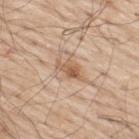– illumination · white-light
– site · the upper back
– patient · male, in their 70s
– lesion size · ≈3.5 mm
– imaging modality · ~15 mm tile from a whole-body skin photo